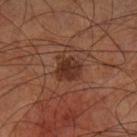notes: imaged on a skin check; not biopsied
site: the left lower leg
image: ~15 mm tile from a whole-body skin photo
TBP lesion metrics: lesion-presence confidence of about 100/100
subject: male, in their 70s
size: ~3 mm (longest diameter)
lighting: cross-polarized illumination